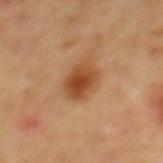Impression:
Part of a total-body skin-imaging series; this lesion was reviewed on a skin check and was not flagged for biopsy.
Image and clinical context:
A male subject, in their mid-60s. The lesion is on the mid back. The lesion's longest dimension is about 4 mm. A 15 mm crop from a total-body photograph taken for skin-cancer surveillance. The tile uses cross-polarized illumination.